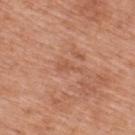lesion size = ≈2.5 mm; tile lighting = white-light illumination; subject = male, aged 68 to 72; site = the upper back; image = 15 mm crop, total-body photography.